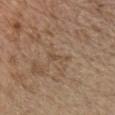The lesion was photographed on a routine skin check and not biopsied; there is no pathology result. The lesion is on the chest. A lesion tile, about 15 mm wide, cut from a 3D total-body photograph. A male subject, about 70 years old.